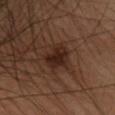workup: catalogued during a skin exam; not biopsied